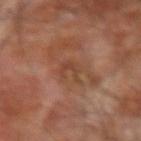- follow-up: imaged on a skin check; not biopsied
- acquisition: ~15 mm crop, total-body skin-cancer survey
- patient: male, about 65 years old
- anatomic site: the right forearm
- lesion diameter: about 3 mm
- lighting: cross-polarized illumination